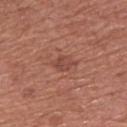About 3 mm across. A male subject in their mid- to late 70s. The tile uses white-light illumination. An algorithmic analysis of the crop reported a classifier nevus-likeness of about 0/100. A 15 mm crop from a total-body photograph taken for skin-cancer surveillance. On the chest.